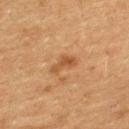notes=total-body-photography surveillance lesion; no biopsy
location=the upper back
automated metrics=a color-variation rating of about 1.5/10 and radial color variation of about 0.5; a nevus-likeness score of about 5/100
patient=female, in their mid- to late 50s
tile lighting=cross-polarized illumination
image source=total-body-photography crop, ~15 mm field of view
size=about 3.5 mm